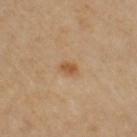Part of a total-body skin-imaging series; this lesion was reviewed on a skin check and was not flagged for biopsy. A close-up tile cropped from a whole-body skin photograph, about 15 mm across. Longest diameter approximately 2 mm. A female patient in their mid-50s. Located on the right upper arm.